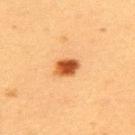Captured during whole-body skin photography for melanoma surveillance; the lesion was not biopsied.